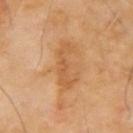biopsy status: catalogued during a skin exam; not biopsied
patient: male, aged 63 to 67
acquisition: ~15 mm tile from a whole-body skin photo
automated lesion analysis: a lesion area of about 9 mm², an eccentricity of roughly 0.95, and a symmetry-axis asymmetry near 0.45; a classifier nevus-likeness of about 0/100
body site: the right upper arm
size: ~5.5 mm (longest diameter)